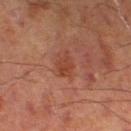Q: What did automated image analysis measure?
A: an area of roughly 5 mm², an eccentricity of roughly 0.75, and a symmetry-axis asymmetry near 0.3; internal color variation of about 2 on a 0–10 scale and a peripheral color-asymmetry measure near 0.5
Q: Who is the patient?
A: male, roughly 70 years of age
Q: Illumination type?
A: cross-polarized
Q: What is the imaging modality?
A: 15 mm crop, total-body photography
Q: Lesion location?
A: the left lower leg